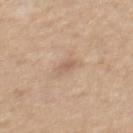Captured during whole-body skin photography for melanoma surveillance; the lesion was not biopsied. A region of skin cropped from a whole-body photographic capture, roughly 15 mm wide. The lesion is on the mid back. Automated image analysis of the tile measured a border-irregularity index near 3/10 and radial color variation of about 0.5. Approximately 2.5 mm at its widest. A female subject, roughly 45 years of age.